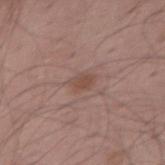{"biopsy_status": "not biopsied; imaged during a skin examination", "patient": {"sex": "male", "age_approx": 55}, "site": "right thigh", "image": {"source": "total-body photography crop", "field_of_view_mm": 15}}